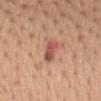Imaged during a routine full-body skin examination; the lesion was not biopsied and no histopathology is available. The lesion is located on the mid back. Cropped from a whole-body photographic skin survey; the tile spans about 15 mm. A female patient, roughly 50 years of age.